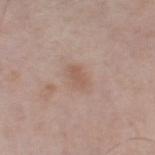<tbp_lesion>
<biopsy_status>not biopsied; imaged during a skin examination</biopsy_status>
<automated_metrics>
  <area_mm2_approx>4.0</area_mm2_approx>
  <eccentricity>0.75</eccentricity>
  <shape_asymmetry>0.3</shape_asymmetry>
  <color_variation_0_10>1.0</color_variation_0_10>
  <nevus_likeness_0_100>0</nevus_likeness_0_100>
  <lesion_detection_confidence_0_100>100</lesion_detection_confidence_0_100>
</automated_metrics>
<lesion_size>
  <long_diameter_mm_approx>2.5</long_diameter_mm_approx>
</lesion_size>
<image>
  <source>total-body photography crop</source>
  <field_of_view_mm>15</field_of_view_mm>
</image>
<lighting>white-light</lighting>
<patient>
  <sex>male</sex>
  <age_approx>75</age_approx>
</patient>
<site>right thigh</site>
</tbp_lesion>